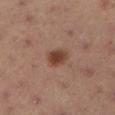body site: the left lower leg; size: ≈3 mm; image: ~15 mm tile from a whole-body skin photo; patient: male, in their mid-50s.